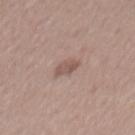  biopsy_status: not biopsied; imaged during a skin examination
  lighting: white-light
  image:
    source: total-body photography crop
    field_of_view_mm: 15
  site: mid back
  automated_metrics:
    area_mm2_approx: 3.5
    eccentricity: 0.75
    nevus_likeness_0_100: 5
    lesion_detection_confidence_0_100: 100
  lesion_size:
    long_diameter_mm_approx: 2.5
  patient:
    sex: male
    age_approx: 55